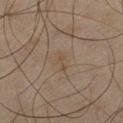This lesion was catalogued during total-body skin photography and was not selected for biopsy. Imaged with cross-polarized lighting. A region of skin cropped from a whole-body photographic capture, roughly 15 mm wide. The patient is a male aged 43–47. On the leg.